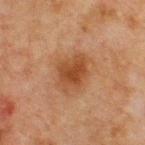Captured during whole-body skin photography for melanoma surveillance; the lesion was not biopsied.
Automated image analysis of the tile measured a shape eccentricity near 0.65 and a symmetry-axis asymmetry near 0.2. It also reported roughly 8 lightness units darker than nearby skin and a normalized lesion–skin contrast near 8. It also reported border irregularity of about 2 on a 0–10 scale, a within-lesion color-variation index near 3.5/10, and a peripheral color-asymmetry measure near 1.
The lesion is located on the front of the torso.
The patient is a male in their mid-60s.
Measured at roughly 4 mm in maximum diameter.
A region of skin cropped from a whole-body photographic capture, roughly 15 mm wide.
Captured under cross-polarized illumination.